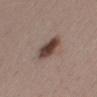The lesion was tiled from a total-body skin photograph and was not biopsied. Automated tile analysis of the lesion measured a footprint of about 7.5 mm², an eccentricity of roughly 0.85, and a symmetry-axis asymmetry near 0.15. The software also gave an average lesion color of about L≈42 a*≈16 b*≈22 (CIELAB). The analysis additionally found a border-irregularity rating of about 1.5/10, a within-lesion color-variation index near 4.5/10, and a peripheral color-asymmetry measure near 1.5. A female subject aged approximately 45. On the mid back. Measured at roughly 4 mm in maximum diameter. A region of skin cropped from a whole-body photographic capture, roughly 15 mm wide.